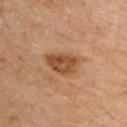Clinical summary:
This image is a 15 mm lesion crop taken from a total-body photograph. The lesion is located on the upper back. A male patient, about 85 years old or older.